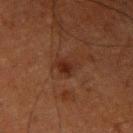biopsy_status: not biopsied; imaged during a skin examination
site: left upper arm
lesion_size:
  long_diameter_mm_approx: 3.0
image:
  source: total-body photography crop
  field_of_view_mm: 15
patient:
  sex: male
  age_approx: 75
lighting: cross-polarized